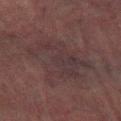workup: imaged on a skin check; not biopsied.